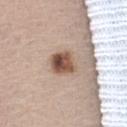The lesion was tiled from a total-body skin photograph and was not biopsied.
A close-up tile cropped from a whole-body skin photograph, about 15 mm across.
A male patient aged approximately 65.
About 3 mm across.
On the abdomen.